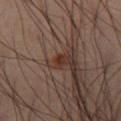  biopsy_status: not biopsied; imaged during a skin examination
  site: right thigh
  patient:
    sex: male
    age_approx: 60
  image:
    source: total-body photography crop
    field_of_view_mm: 15
  lesion_size:
    long_diameter_mm_approx: 1.5